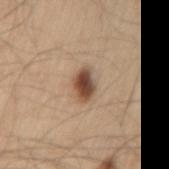  biopsy_status: not biopsied; imaged during a skin examination
  site: left thigh
  lighting: white-light
  image:
    source: total-body photography crop
    field_of_view_mm: 15
  lesion_size:
    long_diameter_mm_approx: 3.5
  patient:
    sex: male
    age_approx: 60
  automated_metrics:
    eccentricity: 0.8
    shape_asymmetry: 0.25
    vs_skin_darker_L: 16.0
    vs_skin_contrast_norm: 11.0
    peripheral_color_asymmetry: 2.0